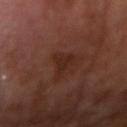Part of a total-body skin-imaging series; this lesion was reviewed on a skin check and was not flagged for biopsy. The lesion is on the right upper arm. A male subject approximately 55 years of age. Imaged with cross-polarized lighting. A 15 mm close-up extracted from a 3D total-body photography capture. The recorded lesion diameter is about 3.5 mm. An algorithmic analysis of the crop reported a footprint of about 4.5 mm², an eccentricity of roughly 0.65, and two-axis asymmetry of about 0.5. The analysis additionally found an average lesion color of about L≈25 a*≈20 b*≈24 (CIELAB), about 6 CIELAB-L* units darker than the surrounding skin, and a normalized lesion–skin contrast near 6.5.